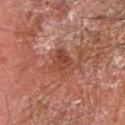Clinical impression:
This lesion was catalogued during total-body skin photography and was not selected for biopsy.
Acquisition and patient details:
The lesion is located on the right forearm. A 15 mm close-up extracted from a 3D total-body photography capture. Imaged with cross-polarized lighting. Longest diameter approximately 3.5 mm. A male patient aged 68–72.